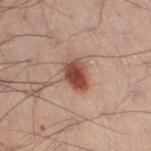Captured during whole-body skin photography for melanoma surveillance; the lesion was not biopsied. A male patient approximately 50 years of age. Cropped from a whole-body photographic skin survey; the tile spans about 15 mm. Located on the right thigh. Longest diameter approximately 3.5 mm. Captured under cross-polarized illumination.